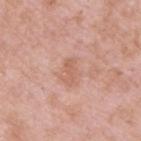Q: Is there a histopathology result?
A: no biopsy performed (imaged during a skin exam)
Q: Who is the patient?
A: male, aged 53 to 57
Q: What is the lesion's diameter?
A: about 3 mm
Q: Where on the body is the lesion?
A: the upper back
Q: How was this image acquired?
A: 15 mm crop, total-body photography
Q: Automated lesion metrics?
A: a footprint of about 3 mm², an outline eccentricity of about 0.95 (0 = round, 1 = elongated), and a shape-asymmetry score of about 0.25 (0 = symmetric); a nevus-likeness score of about 0/100 and a lesion-detection confidence of about 100/100